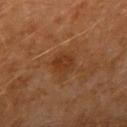| feature | finding |
|---|---|
| workup | total-body-photography surveillance lesion; no biopsy |
| acquisition | ~15 mm crop, total-body skin-cancer survey |
| anatomic site | the right upper arm |
| automated metrics | an area of roughly 5.5 mm², a shape eccentricity near 0.4, and two-axis asymmetry of about 0.15; an average lesion color of about L≈33 a*≈21 b*≈32 (CIELAB) and a normalized lesion–skin contrast near 6.5 |
| size | ≈3 mm |
| subject | male, in their 60s |